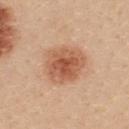<tbp_lesion>
<biopsy_status>not biopsied; imaged during a skin examination</biopsy_status>
<image>
  <source>total-body photography crop</source>
  <field_of_view_mm>15</field_of_view_mm>
</image>
<automated_metrics>
  <vs_skin_darker_L>12.0</vs_skin_darker_L>
  <nevus_likeness_0_100>100</nevus_likeness_0_100>
  <lesion_detection_confidence_0_100>100</lesion_detection_confidence_0_100>
</automated_metrics>
<site>back</site>
<lighting>white-light</lighting>
<lesion_size>
  <long_diameter_mm_approx>5.0</long_diameter_mm_approx>
</lesion_size>
<patient>
  <sex>female</sex>
  <age_approx>25</age_approx>
</patient>
</tbp_lesion>